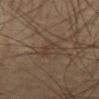Impression:
Recorded during total-body skin imaging; not selected for excision or biopsy.
Context:
On the abdomen. The lesion-visualizer software estimated a mean CIELAB color near L≈37 a*≈13 b*≈23 and a lesion-to-skin contrast of about 4.5 (normalized; higher = more distinct). The analysis additionally found a border-irregularity rating of about 5.5/10 and a color-variation rating of about 0/10. And it measured a nevus-likeness score of about 0/100. Captured under cross-polarized illumination. Cropped from a total-body skin-imaging series; the visible field is about 15 mm. The subject is a male aged 58–62. Approximately 3 mm at its widest.